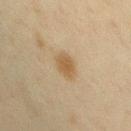Assessment: The lesion was tiled from a total-body skin photograph and was not biopsied. Clinical summary: On the chest. A lesion tile, about 15 mm wide, cut from a 3D total-body photograph. Captured under cross-polarized illumination. A female subject aged approximately 40.